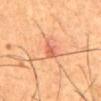The lesion was tiled from a total-body skin photograph and was not biopsied.
A male patient roughly 55 years of age.
The lesion-visualizer software estimated an average lesion color of about L≈57 a*≈28 b*≈35 (CIELAB), roughly 10 lightness units darker than nearby skin, and a normalized border contrast of about 6.5.
A lesion tile, about 15 mm wide, cut from a 3D total-body photograph.
From the abdomen.
Captured under cross-polarized illumination.